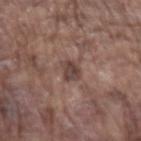  biopsy_status: not biopsied; imaged during a skin examination
  automated_metrics:
    color_variation_0_10: 3.0
    peripheral_color_asymmetry: 1.0
  lesion_size:
    long_diameter_mm_approx: 2.5
  image:
    source: total-body photography crop
    field_of_view_mm: 15
  site: left forearm
  patient:
    sex: male
    age_approx: 75